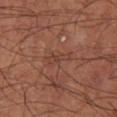{"biopsy_status": "not biopsied; imaged during a skin examination"}